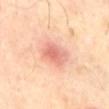Longest diameter approximately 3.5 mm. This is a cross-polarized tile. From the abdomen. Cropped from a total-body skin-imaging series; the visible field is about 15 mm. Automated image analysis of the tile measured a footprint of about 7.5 mm², an outline eccentricity of about 0.7 (0 = round, 1 = elongated), and a shape-asymmetry score of about 0.15 (0 = symmetric). It also reported about 11 CIELAB-L* units darker than the surrounding skin and a normalized lesion–skin contrast near 6.5. The subject is a male in their 70s.